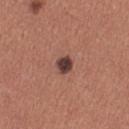Q: Was this lesion biopsied?
A: no biopsy performed (imaged during a skin exam)
Q: What is the anatomic site?
A: the right thigh
Q: What did automated image analysis measure?
A: a lesion color around L≈40 a*≈20 b*≈22 in CIELAB and roughly 15 lightness units darker than nearby skin; border irregularity of about 1.5 on a 0–10 scale, internal color variation of about 2.5 on a 0–10 scale, and radial color variation of about 1; a classifier nevus-likeness of about 65/100 and a detector confidence of about 100 out of 100 that the crop contains a lesion
Q: How was this image acquired?
A: ~15 mm crop, total-body skin-cancer survey
Q: How was the tile lit?
A: white-light illumination
Q: What is the lesion's diameter?
A: ≈2 mm
Q: Patient demographics?
A: female, aged approximately 35